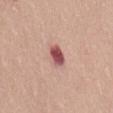Captured during whole-body skin photography for melanoma surveillance; the lesion was not biopsied.
On the lower back.
The patient is a female approximately 50 years of age.
The lesion-visualizer software estimated a border-irregularity index near 1.5/10, a within-lesion color-variation index near 5/10, and a peripheral color-asymmetry measure near 1.5.
Captured under white-light illumination.
A 15 mm close-up tile from a total-body photography series done for melanoma screening.